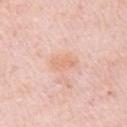Findings:
- subject · female, roughly 65 years of age
- image source · ~15 mm tile from a whole-body skin photo
- lesion size · ≈3 mm
- body site · the right upper arm